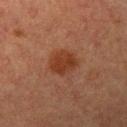Q: Was a biopsy performed?
A: imaged on a skin check; not biopsied
Q: Lesion location?
A: the left upper arm
Q: What are the patient's age and sex?
A: female, in their mid-50s
Q: What did automated image analysis measure?
A: a border-irregularity rating of about 2.5/10, a color-variation rating of about 2/10, and radial color variation of about 0.5; a lesion-detection confidence of about 100/100
Q: What lighting was used for the tile?
A: cross-polarized illumination
Q: What kind of image is this?
A: ~15 mm tile from a whole-body skin photo
Q: What is the lesion's diameter?
A: about 3 mm